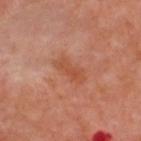Recorded during total-body skin imaging; not selected for excision or biopsy. Measured at roughly 4 mm in maximum diameter. Automated tile analysis of the lesion measured a lesion color around L≈50 a*≈27 b*≈34 in CIELAB, roughly 7 lightness units darker than nearby skin, and a normalized lesion–skin contrast near 6. And it measured border irregularity of about 3 on a 0–10 scale and a peripheral color-asymmetry measure near 1. It also reported a nevus-likeness score of about 0/100 and a detector confidence of about 100 out of 100 that the crop contains a lesion. The lesion is located on the upper back. The tile uses cross-polarized illumination. A 15 mm close-up extracted from a 3D total-body photography capture. A male patient aged 48 to 52.